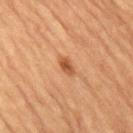Q: Was this lesion biopsied?
A: catalogued during a skin exam; not biopsied
Q: What lighting was used for the tile?
A: cross-polarized illumination
Q: What is the imaging modality?
A: ~15 mm crop, total-body skin-cancer survey
Q: How large is the lesion?
A: about 2.5 mm
Q: What is the anatomic site?
A: the lower back
Q: What are the patient's age and sex?
A: male, aged approximately 85 or older